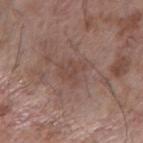biopsy_status: not biopsied; imaged during a skin examination
site: right forearm
image:
  source: total-body photography crop
  field_of_view_mm: 15
patient:
  sex: male
  age_approx: 60